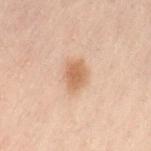Recorded during total-body skin imaging; not selected for excision or biopsy. This image is a 15 mm lesion crop taken from a total-body photograph. An algorithmic analysis of the crop reported a lesion area of about 7 mm² and a shape eccentricity near 0.55. The software also gave a lesion color around L≈50 a*≈17 b*≈29 in CIELAB, about 9 CIELAB-L* units darker than the surrounding skin, and a normalized border contrast of about 7.5. And it measured a lesion-detection confidence of about 100/100. The lesion is located on the left thigh. About 3 mm across. The patient is a male aged approximately 40. The tile uses cross-polarized illumination.